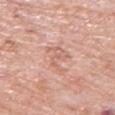The lesion was tiled from a total-body skin photograph and was not biopsied. A close-up tile cropped from a whole-body skin photograph, about 15 mm across. About 4.5 mm across. An algorithmic analysis of the crop reported a shape eccentricity near 0.85 and a symmetry-axis asymmetry near 0.6. And it measured a mean CIELAB color near L≈64 a*≈23 b*≈29 and a normalized lesion–skin contrast near 4.5. It also reported a nevus-likeness score of about 0/100 and a detector confidence of about 100 out of 100 that the crop contains a lesion. On the arm. Captured under white-light illumination. The patient is a male about 80 years old.